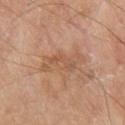size: about 5.5 mm
subject: male, about 80 years old
image: total-body-photography crop, ~15 mm field of view
automated metrics: a footprint of about 9 mm², an outline eccentricity of about 0.95 (0 = round, 1 = elongated), and two-axis asymmetry of about 0.35; an average lesion color of about L≈56 a*≈21 b*≈33 (CIELAB), a lesion–skin lightness drop of about 7, and a lesion-to-skin contrast of about 5 (normalized; higher = more distinct); border irregularity of about 5 on a 0–10 scale, a color-variation rating of about 2.5/10, and peripheral color asymmetry of about 1; an automated nevus-likeness rating near 0 out of 100
tile lighting: white-light
location: the upper back